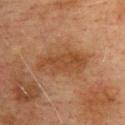| key | value |
|---|---|
| imaging modality | total-body-photography crop, ~15 mm field of view |
| TBP lesion metrics | a footprint of about 14 mm², a shape eccentricity near 0.9, and a shape-asymmetry score of about 0.15 (0 = symmetric); a lesion–skin lightness drop of about 7 and a normalized lesion–skin contrast near 7; an automated nevus-likeness rating near 0 out of 100 and a detector confidence of about 100 out of 100 that the crop contains a lesion |
| site | the upper back |
| subject | male, roughly 75 years of age |
| tile lighting | cross-polarized |
| diameter | ≈6.5 mm |